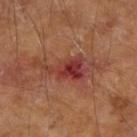  patient:
    sex: male
    age_approx: 65
  image:
    source: total-body photography crop
    field_of_view_mm: 15
  site: right upper arm
  lighting: cross-polarized
  lesion_size:
    long_diameter_mm_approx: 7.5